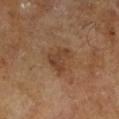| field | value |
|---|---|
| follow-up | catalogued during a skin exam; not biopsied |
| subject | male, in their mid-60s |
| site | the left lower leg |
| diameter | ≈4 mm |
| tile lighting | cross-polarized |
| acquisition | 15 mm crop, total-body photography |
| automated lesion analysis | a border-irregularity index near 4/10 and a peripheral color-asymmetry measure near 1 |